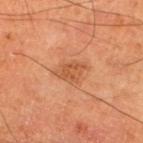Findings:
- notes — imaged on a skin check; not biopsied
- patient — male, roughly 60 years of age
- image source — ~15 mm tile from a whole-body skin photo
- location — the left lower leg